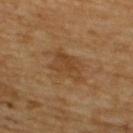Recorded during total-body skin imaging; not selected for excision or biopsy.
A male subject in their 60s.
A region of skin cropped from a whole-body photographic capture, roughly 15 mm wide.
The total-body-photography lesion software estimated a lesion area of about 10 mm², an outline eccentricity of about 0.7 (0 = round, 1 = elongated), and a shape-asymmetry score of about 0.3 (0 = symmetric). It also reported a lesion–skin lightness drop of about 7 and a lesion-to-skin contrast of about 6 (normalized; higher = more distinct). And it measured a border-irregularity index near 4/10, a color-variation rating of about 3/10, and a peripheral color-asymmetry measure near 1.
Approximately 4.5 mm at its widest.
The lesion is on the upper back.
Captured under cross-polarized illumination.